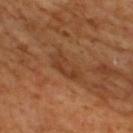{
  "biopsy_status": "not biopsied; imaged during a skin examination",
  "lighting": "cross-polarized",
  "automated_metrics": {
    "area_mm2_approx": 7.0,
    "eccentricity": 0.9,
    "shape_asymmetry": 0.25,
    "cielab_L": 38,
    "cielab_a": 22,
    "cielab_b": 33,
    "vs_skin_darker_L": 6.0,
    "lesion_detection_confidence_0_100": 95
  },
  "lesion_size": {
    "long_diameter_mm_approx": 4.5
  },
  "image": {
    "source": "total-body photography crop",
    "field_of_view_mm": 15
  },
  "patient": {
    "sex": "male",
    "age_approx": 65
  },
  "site": "upper back"
}